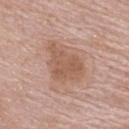– follow-up: catalogued during a skin exam; not biopsied
– subject: female, aged 63–67
– body site: the back
– image: total-body-photography crop, ~15 mm field of view
– diameter: ~6 mm (longest diameter)
– tile lighting: white-light
– automated metrics: a border-irregularity index near 3.5/10, internal color variation of about 2.5 on a 0–10 scale, and peripheral color asymmetry of about 1